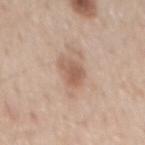Clinical impression: Part of a total-body skin-imaging series; this lesion was reviewed on a skin check and was not flagged for biopsy. Image and clinical context: A 15 mm close-up tile from a total-body photography series done for melanoma screening. Located on the mid back. The patient is a male roughly 60 years of age.